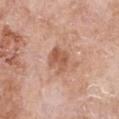Captured during whole-body skin photography for melanoma surveillance; the lesion was not biopsied.
A female subject, aged 73–77.
The lesion is located on the chest.
A 15 mm crop from a total-body photograph taken for skin-cancer surveillance.